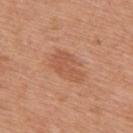The lesion is on the upper back. This image is a 15 mm lesion crop taken from a total-body photograph. The patient is a male roughly 70 years of age.